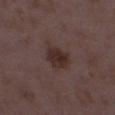Q: Is there a histopathology result?
A: total-body-photography surveillance lesion; no biopsy
Q: What is the imaging modality?
A: 15 mm crop, total-body photography
Q: What lighting was used for the tile?
A: white-light illumination
Q: Patient demographics?
A: female, aged 33 to 37
Q: Where on the body is the lesion?
A: the left thigh